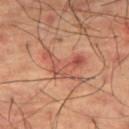Q: Is there a histopathology result?
A: no biopsy performed (imaged during a skin exam)
Q: What did automated image analysis measure?
A: a mean CIELAB color near L≈53 a*≈28 b*≈31, about 8 CIELAB-L* units darker than the surrounding skin, and a normalized border contrast of about 6; a border-irregularity index near 9.5/10, a color-variation rating of about 5/10, and radial color variation of about 0.5; a classifier nevus-likeness of about 0/100 and lesion-presence confidence of about 100/100
Q: Lesion size?
A: about 5 mm
Q: What is the imaging modality?
A: total-body-photography crop, ~15 mm field of view
Q: What lighting was used for the tile?
A: cross-polarized illumination
Q: What are the patient's age and sex?
A: male, aged 63–67
Q: Lesion location?
A: the right thigh